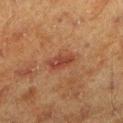follow-up = imaged on a skin check; not biopsied | diameter = ≈3.5 mm | subject = male, aged 58–62 | body site = the leg | tile lighting = cross-polarized illumination | automated lesion analysis = a lesion color around L≈33 a*≈22 b*≈26 in CIELAB, roughly 8 lightness units darker than nearby skin, and a normalized lesion–skin contrast near 7.5; an automated nevus-likeness rating near 85 out of 100 and a detector confidence of about 100 out of 100 that the crop contains a lesion | image = ~15 mm crop, total-body skin-cancer survey.